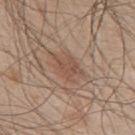Q: Was this lesion biopsied?
A: total-body-photography surveillance lesion; no biopsy
Q: What is the imaging modality?
A: total-body-photography crop, ~15 mm field of view
Q: Where on the body is the lesion?
A: the upper back
Q: Who is the patient?
A: male, aged 48 to 52